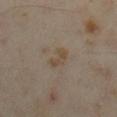notes = total-body-photography surveillance lesion; no biopsy
imaging modality = ~15 mm crop, total-body skin-cancer survey
body site = the leg
subject = female, aged approximately 35